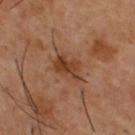This lesion was catalogued during total-body skin photography and was not selected for biopsy.
Approximately 4 mm at its widest.
The tile uses cross-polarized illumination.
The lesion is located on the back.
The subject is a male about 55 years old.
Automated image analysis of the tile measured a lesion area of about 6.5 mm² and an outline eccentricity of about 0.8 (0 = round, 1 = elongated). And it measured a classifier nevus-likeness of about 0/100 and a detector confidence of about 100 out of 100 that the crop contains a lesion.
This image is a 15 mm lesion crop taken from a total-body photograph.